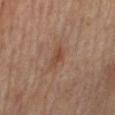Q: Is there a histopathology result?
A: no biopsy performed (imaged during a skin exam)
Q: Who is the patient?
A: female, aged 63–67
Q: Where on the body is the lesion?
A: the right leg
Q: How was this image acquired?
A: ~15 mm crop, total-body skin-cancer survey
Q: Illumination type?
A: cross-polarized illumination
Q: Lesion size?
A: about 2.5 mm